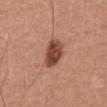Impression:
Recorded during total-body skin imaging; not selected for excision or biopsy.
Acquisition and patient details:
On the back. A lesion tile, about 15 mm wide, cut from a 3D total-body photograph. About 4 mm across. Imaged with white-light lighting. A male patient, in their mid-40s.